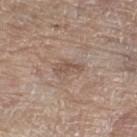Case summary:
• workup — no biopsy performed (imaged during a skin exam)
• diameter — ≈3 mm
• anatomic site — the leg
• image source — total-body-photography crop, ~15 mm field of view
• subject — female, roughly 75 years of age